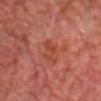Clinical impression:
The lesion was tiled from a total-body skin photograph and was not biopsied.